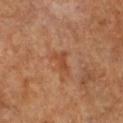notes=total-body-photography surveillance lesion; no biopsy | acquisition=total-body-photography crop, ~15 mm field of view | subject=female, aged around 60 | automated lesion analysis=an average lesion color of about L≈43 a*≈24 b*≈33 (CIELAB) | site=the front of the torso.